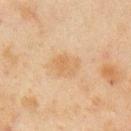Impression:
No biopsy was performed on this lesion — it was imaged during a full skin examination and was not determined to be concerning.
Image and clinical context:
A 15 mm close-up extracted from a 3D total-body photography capture. A male patient, in their mid- to late 40s. Located on the right upper arm.